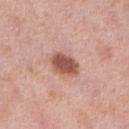A female patient about 40 years old.
Located on the right lower leg.
Captured under white-light illumination.
Cropped from a total-body skin-imaging series; the visible field is about 15 mm.
Automated tile analysis of the lesion measured an area of roughly 7.5 mm². The software also gave a normalized border contrast of about 10. And it measured a classifier nevus-likeness of about 95/100 and a lesion-detection confidence of about 100/100.